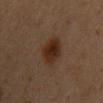biopsy_status: not biopsied; imaged during a skin examination
patient:
  sex: female
  age_approx: 45
lighting: cross-polarized
site: chest
automated_metrics:
  area_mm2_approx: 10.0
  eccentricity: 0.65
  shape_asymmetry: 0.15
image:
  source: total-body photography crop
  field_of_view_mm: 15
lesion_size:
  long_diameter_mm_approx: 4.0